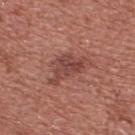follow-up: imaged on a skin check; not biopsied | lighting: white-light | subject: male, in their mid-40s | image source: 15 mm crop, total-body photography | anatomic site: the front of the torso | automated lesion analysis: an average lesion color of about L≈45 a*≈25 b*≈25 (CIELAB), about 9 CIELAB-L* units darker than the surrounding skin, and a normalized lesion–skin contrast near 7; a nevus-likeness score of about 5/100 and a lesion-detection confidence of about 100/100.